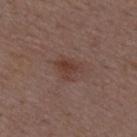A region of skin cropped from a whole-body photographic capture, roughly 15 mm wide. Located on the mid back. A male subject, aged approximately 50.